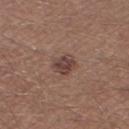{
  "biopsy_status": "not biopsied; imaged during a skin examination",
  "automated_metrics": {
    "vs_skin_contrast_norm": 8.5,
    "border_irregularity_0_10": 2.5,
    "color_variation_0_10": 2.5,
    "peripheral_color_asymmetry": 1.0,
    "nevus_likeness_0_100": 25
  },
  "patient": {
    "sex": "male",
    "age_approx": 40
  },
  "image": {
    "source": "total-body photography crop",
    "field_of_view_mm": 15
  },
  "lighting": "white-light",
  "site": "right thigh",
  "lesion_size": {
    "long_diameter_mm_approx": 3.0
  }
}